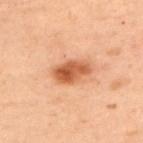Clinical summary:
Longest diameter approximately 4 mm. A 15 mm crop from a total-body photograph taken for skin-cancer surveillance. The subject is a female in their mid- to late 50s. The lesion is located on the back.